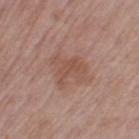The lesion-visualizer software estimated a border-irregularity rating of about 5/10 and radial color variation of about 1. The analysis additionally found a nevus-likeness score of about 0/100 and lesion-presence confidence of about 100/100. A female patient, aged around 65. From the left thigh. About 4 mm across. A close-up tile cropped from a whole-body skin photograph, about 15 mm across. Imaged with white-light lighting.